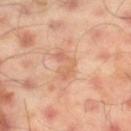Q: Is there a histopathology result?
A: no biopsy performed (imaged during a skin exam)
Q: Automated lesion metrics?
A: a symmetry-axis asymmetry near 0.5; an average lesion color of about L≈63 a*≈23 b*≈34 (CIELAB) and a normalized lesion–skin contrast near 5; peripheral color asymmetry of about 1; a classifier nevus-likeness of about 0/100 and lesion-presence confidence of about 100/100
Q: What is the anatomic site?
A: the leg
Q: How was this image acquired?
A: 15 mm crop, total-body photography
Q: What are the patient's age and sex?
A: male, about 45 years old